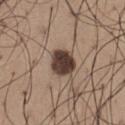follow-up — total-body-photography surveillance lesion; no biopsy.